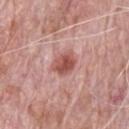Clinical impression: Recorded during total-body skin imaging; not selected for excision or biopsy. Background: A male subject aged around 70. Imaged with white-light lighting. The total-body-photography lesion software estimated a lesion area of about 6.5 mm², a shape eccentricity near 0.65, and two-axis asymmetry of about 0.25. The software also gave a lesion color around L≈53 a*≈27 b*≈25 in CIELAB and a lesion–skin lightness drop of about 13. It also reported a border-irregularity rating of about 2.5/10, a color-variation rating of about 4/10, and peripheral color asymmetry of about 1.5. The software also gave an automated nevus-likeness rating near 70 out of 100 and a detector confidence of about 100 out of 100 that the crop contains a lesion. A 15 mm close-up tile from a total-body photography series done for melanoma screening. Measured at roughly 3 mm in maximum diameter. The lesion is located on the chest.